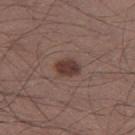biopsy_status: not biopsied; imaged during a skin examination
lesion_size:
  long_diameter_mm_approx: 3.0
lighting: white-light
site: left thigh
image:
  source: total-body photography crop
  field_of_view_mm: 15
patient:
  sex: male
  age_approx: 55